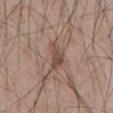• notes · total-body-photography surveillance lesion; no biopsy
• body site · the abdomen
• subject · male, aged around 65
• size · about 3.5 mm
• tile lighting · white-light illumination
• acquisition · ~15 mm crop, total-body skin-cancer survey